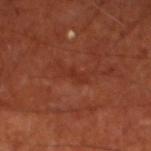{"biopsy_status": "not biopsied; imaged during a skin examination", "image": {"source": "total-body photography crop", "field_of_view_mm": 15}, "site": "left lower leg", "patient": {"sex": "male", "age_approx": 70}, "lighting": "cross-polarized", "lesion_size": {"long_diameter_mm_approx": 2.5}}